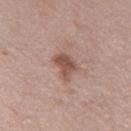Captured under white-light illumination.
On the left thigh.
Automated image analysis of the tile measured border irregularity of about 3 on a 0–10 scale, a color-variation rating of about 3/10, and radial color variation of about 1. It also reported a classifier nevus-likeness of about 30/100 and a lesion-detection confidence of about 100/100.
A lesion tile, about 15 mm wide, cut from a 3D total-body photograph.
A female patient, aged approximately 40.